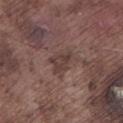Recorded during total-body skin imaging; not selected for excision or biopsy. The tile uses white-light illumination. A male subject, aged approximately 75. A roughly 15 mm field-of-view crop from a total-body skin photograph. From the left lower leg. Measured at roughly 3 mm in maximum diameter.